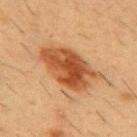Captured during whole-body skin photography for melanoma surveillance; the lesion was not biopsied.
Longest diameter approximately 7 mm.
The lesion is located on the chest.
The tile uses cross-polarized illumination.
Cropped from a whole-body photographic skin survey; the tile spans about 15 mm.
A male patient, aged 53 to 57.